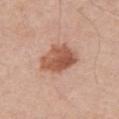notes = imaged on a skin check; not biopsied | anatomic site = the chest | TBP lesion metrics = a mean CIELAB color near L≈55 a*≈23 b*≈31 and roughly 13 lightness units darker than nearby skin; a classifier nevus-likeness of about 85/100 | lesion size = ≈5 mm | lighting = white-light | subject = male, aged 68 to 72 | image = 15 mm crop, total-body photography.